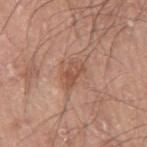notes: catalogued during a skin exam; not biopsied | anatomic site: the left arm | subject: male, aged approximately 70 | image: ~15 mm crop, total-body skin-cancer survey.